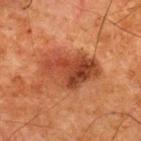{
  "biopsy_status": "not biopsied; imaged during a skin examination"
}